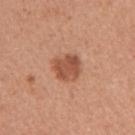Impression: Recorded during total-body skin imaging; not selected for excision or biopsy. Context: Longest diameter approximately 3.5 mm. A close-up tile cropped from a whole-body skin photograph, about 15 mm across. Located on the arm. A female subject, aged 33 to 37. This is a white-light tile.